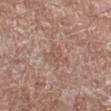The lesion was tiled from a total-body skin photograph and was not biopsied. This is a white-light tile. On the left lower leg. Cropped from a whole-body photographic skin survey; the tile spans about 15 mm. Automated tile analysis of the lesion measured an area of roughly 5.5 mm², an outline eccentricity of about 0.55 (0 = round, 1 = elongated), and a symmetry-axis asymmetry near 0.55. And it measured about 6 CIELAB-L* units darker than the surrounding skin and a normalized border contrast of about 4.5. A male subject aged 58 to 62.